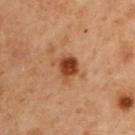• biopsy status · total-body-photography surveillance lesion; no biopsy
• location · the right upper arm
• tile lighting · cross-polarized illumination
• patient · male, approximately 60 years of age
• size · ~3 mm (longest diameter)
• image source · 15 mm crop, total-body photography